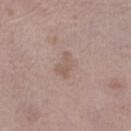Assessment: The lesion was tiled from a total-body skin photograph and was not biopsied. Clinical summary: The lesion is located on the right lower leg. The patient is a female aged 63 to 67. Cropped from a whole-body photographic skin survey; the tile spans about 15 mm.